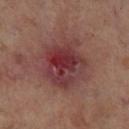Q: Was a biopsy performed?
A: total-body-photography surveillance lesion; no biopsy
Q: Where on the body is the lesion?
A: the left lower leg
Q: What kind of image is this?
A: total-body-photography crop, ~15 mm field of view
Q: How large is the lesion?
A: ~6 mm (longest diameter)
Q: Illumination type?
A: cross-polarized
Q: Who is the patient?
A: male, approximately 70 years of age